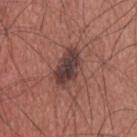follow-up: total-body-photography surveillance lesion; no biopsy | image source: total-body-photography crop, ~15 mm field of view | body site: the upper back | diameter: ~4.5 mm (longest diameter) | patient: male, aged 33–37 | automated metrics: an area of roughly 11 mm², an eccentricity of roughly 0.8, and a shape-asymmetry score of about 0.2 (0 = symmetric); an average lesion color of about L≈39 a*≈19 b*≈20 (CIELAB), a lesion–skin lightness drop of about 12, and a normalized lesion–skin contrast near 10.5; border irregularity of about 2.5 on a 0–10 scale and radial color variation of about 1.5 | illumination: white-light.